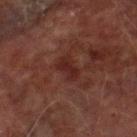  biopsy_status: not biopsied; imaged during a skin examination
  patient:
    sex: male
    age_approx: 75
  lesion_size:
    long_diameter_mm_approx: 3.0
  site: right lower leg
  lighting: cross-polarized
  image:
    source: total-body photography crop
    field_of_view_mm: 15
  automated_metrics:
    nevus_likeness_0_100: 60
    lesion_detection_confidence_0_100: 100